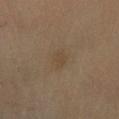The lesion was tiled from a total-body skin photograph and was not biopsied.
From the right lower leg.
Cropped from a whole-body photographic skin survey; the tile spans about 15 mm.
The lesion's longest dimension is about 3 mm.
A female patient, in their 70s.
Captured under cross-polarized illumination.
The total-body-photography lesion software estimated an average lesion color of about L≈45 a*≈12 b*≈29 (CIELAB), a lesion–skin lightness drop of about 5, and a normalized border contrast of about 4.5. The analysis additionally found border irregularity of about 2.5 on a 0–10 scale, a within-lesion color-variation index near 1.5/10, and peripheral color asymmetry of about 0.5. The software also gave a nevus-likeness score of about 5/100 and a lesion-detection confidence of about 100/100.